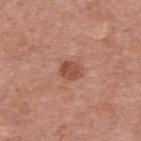Impression:
The lesion was photographed on a routine skin check and not biopsied; there is no pathology result.
Clinical summary:
The lesion-visualizer software estimated a footprint of about 4.5 mm², an outline eccentricity of about 0.55 (0 = round, 1 = elongated), and a symmetry-axis asymmetry near 0.2. The software also gave lesion-presence confidence of about 100/100. Captured under white-light illumination. The subject is a female approximately 70 years of age. A 15 mm close-up extracted from a 3D total-body photography capture. The lesion is located on the back. Longest diameter approximately 2.5 mm.